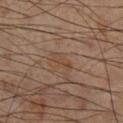Notes:
* workup — total-body-photography surveillance lesion; no biopsy